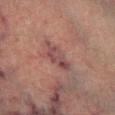| field | value |
|---|---|
| notes | no biopsy performed (imaged during a skin exam) |
| image source | total-body-photography crop, ~15 mm field of view |
| diameter | about 5 mm |
| patient | male, aged approximately 75 |
| location | the right lower leg |
| illumination | cross-polarized |